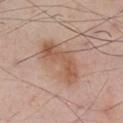| key | value |
|---|---|
| workup | total-body-photography surveillance lesion; no biopsy |
| image source | total-body-photography crop, ~15 mm field of view |
| lesion diameter | ~6 mm (longest diameter) |
| anatomic site | the chest |
| subject | male, aged 58–62 |